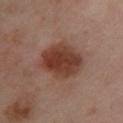Clinical impression: Captured during whole-body skin photography for melanoma surveillance; the lesion was not biopsied. Clinical summary: Longest diameter approximately 5 mm. The lesion is located on the left lower leg. A female subject in their mid- to late 50s. Captured under cross-polarized illumination. An algorithmic analysis of the crop reported a lesion color around L≈39 a*≈22 b*≈27 in CIELAB, about 12 CIELAB-L* units darker than the surrounding skin, and a normalized border contrast of about 10.5. The software also gave a color-variation rating of about 5/10 and a peripheral color-asymmetry measure near 1.5. And it measured a lesion-detection confidence of about 100/100. A roughly 15 mm field-of-view crop from a total-body skin photograph.